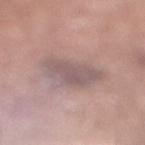| key | value |
|---|---|
| follow-up | no biopsy performed (imaged during a skin exam) |
| imaging modality | ~15 mm crop, total-body skin-cancer survey |
| body site | the right lower leg |
| subject | male, aged 68–72 |
| diameter | about 5.5 mm |
| TBP lesion metrics | border irregularity of about 3 on a 0–10 scale, a within-lesion color-variation index near 2.5/10, and a peripheral color-asymmetry measure near 1; a classifier nevus-likeness of about 0/100 and a lesion-detection confidence of about 75/100 |
| illumination | white-light illumination |